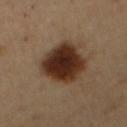| key | value |
|---|---|
| notes | imaged on a skin check; not biopsied |
| diameter | about 6 mm |
| subject | male, approximately 50 years of age |
| image source | 15 mm crop, total-body photography |
| illumination | cross-polarized |
| site | the abdomen |